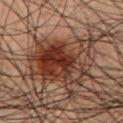Context: A close-up tile cropped from a whole-body skin photograph, about 15 mm across. Automated image analysis of the tile measured a lesion area of about 34 mm², an eccentricity of roughly 0.65, and two-axis asymmetry of about 0.35. The software also gave a border-irregularity index near 5/10 and a within-lesion color-variation index near 9.5/10. It also reported an automated nevus-likeness rating near 65 out of 100 and a lesion-detection confidence of about 100/100. The recorded lesion diameter is about 8 mm. The patient is a male aged around 50. The lesion is on the chest.